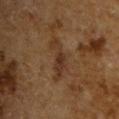Clinical impression:
Captured during whole-body skin photography for melanoma surveillance; the lesion was not biopsied.
Background:
The subject is a male aged 63 to 67. An algorithmic analysis of the crop reported a lesion area of about 7.5 mm² and an eccentricity of roughly 0.9. The software also gave a lesion–skin lightness drop of about 6. The software also gave an automated nevus-likeness rating near 0 out of 100. Longest diameter approximately 5 mm. The lesion is located on the upper back. A 15 mm crop from a total-body photograph taken for skin-cancer surveillance. Captured under cross-polarized illumination.